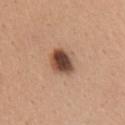<case>
  <biopsy_status>not biopsied; imaged during a skin examination</biopsy_status>
  <lighting>white-light</lighting>
  <site>chest</site>
  <patient>
    <sex>female</sex>
    <age_approx>40</age_approx>
  </patient>
  <automated_metrics>
    <peripheral_color_asymmetry>2.5</peripheral_color_asymmetry>
  </automated_metrics>
  <lesion_size>
    <long_diameter_mm_approx>4.0</long_diameter_mm_approx>
  </lesion_size>
  <image>
    <source>total-body photography crop</source>
    <field_of_view_mm>15</field_of_view_mm>
  </image>
</case>